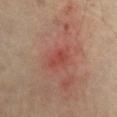Clinical impression:
Recorded during total-body skin imaging; not selected for excision or biopsy.
Acquisition and patient details:
Longest diameter approximately 3 mm. The lesion-visualizer software estimated roughly 6 lightness units darker than nearby skin. The analysis additionally found a color-variation rating of about 2.5/10 and a peripheral color-asymmetry measure near 1. The lesion is on the chest. The patient is a male approximately 70 years of age. A region of skin cropped from a whole-body photographic capture, roughly 15 mm wide. This is a cross-polarized tile.